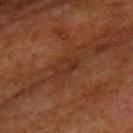Q: Who is the patient?
A: male, aged 63 to 67
Q: How was the tile lit?
A: cross-polarized
Q: How large is the lesion?
A: ~3 mm (longest diameter)
Q: Where on the body is the lesion?
A: the upper back
Q: What is the imaging modality?
A: total-body-photography crop, ~15 mm field of view
Q: Automated lesion metrics?
A: a lesion color around L≈30 a*≈22 b*≈29 in CIELAB; border irregularity of about 2.5 on a 0–10 scale and radial color variation of about 1; an automated nevus-likeness rating near 0 out of 100 and a lesion-detection confidence of about 95/100